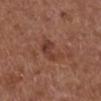notes = imaged on a skin check; not biopsied | tile lighting = white-light | anatomic site = the right lower leg | patient = male, aged 73 to 77 | acquisition = total-body-photography crop, ~15 mm field of view.